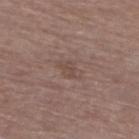Captured during whole-body skin photography for melanoma surveillance; the lesion was not biopsied. From the leg. This image is a 15 mm lesion crop taken from a total-body photograph. The subject is a male roughly 65 years of age.